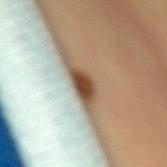Impression: Part of a total-body skin-imaging series; this lesion was reviewed on a skin check and was not flagged for biopsy. Clinical summary: The lesion is located on the right thigh. The subject is a male aged approximately 50. Cropped from a total-body skin-imaging series; the visible field is about 15 mm.